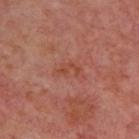The lesion was tiled from a total-body skin photograph and was not biopsied. A lesion tile, about 15 mm wide, cut from a 3D total-body photograph. The patient is in their mid-50s. The lesion is located on the upper back.